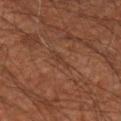biopsy_status: not biopsied; imaged during a skin examination
lighting: cross-polarized
site: right upper arm
image:
  source: total-body photography crop
  field_of_view_mm: 15
patient:
  sex: male
  age_approx: 45